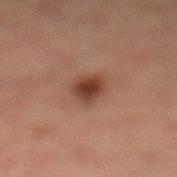Clinical impression:
The lesion was photographed on a routine skin check and not biopsied; there is no pathology result.
Context:
The lesion is on the left lower leg. Automated image analysis of the tile measured a lesion color around L≈33 a*≈19 b*≈24 in CIELAB, about 10 CIELAB-L* units darker than the surrounding skin, and a normalized border contrast of about 9.5. And it measured border irregularity of about 1.5 on a 0–10 scale, internal color variation of about 3.5 on a 0–10 scale, and peripheral color asymmetry of about 1. Longest diameter approximately 3 mm. This is a cross-polarized tile. A lesion tile, about 15 mm wide, cut from a 3D total-body photograph. A male patient aged 28–32.